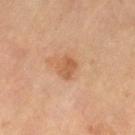Context:
A male subject approximately 65 years of age. A close-up tile cropped from a whole-body skin photograph, about 15 mm across. Located on the left thigh. The lesion-visualizer software estimated an area of roughly 5 mm², an eccentricity of roughly 0.6, and two-axis asymmetry of about 0.2. It also reported border irregularity of about 1.5 on a 0–10 scale, internal color variation of about 2.5 on a 0–10 scale, and a peripheral color-asymmetry measure near 1. And it measured a classifier nevus-likeness of about 40/100 and a lesion-detection confidence of about 100/100. This is a cross-polarized tile.